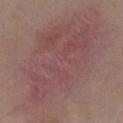Captured under white-light illumination.
An algorithmic analysis of the crop reported an area of roughly 90 mm², an outline eccentricity of about 0.85 (0 = round, 1 = elongated), and a symmetry-axis asymmetry near 0.25. It also reported a border-irregularity rating of about 5/10, internal color variation of about 4.5 on a 0–10 scale, and a peripheral color-asymmetry measure near 1.5.
From the chest.
Longest diameter approximately 15 mm.
The patient is a female about 35 years old.
Cropped from a whole-body photographic skin survey; the tile spans about 15 mm.